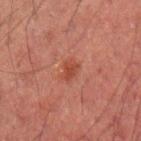Assessment: Part of a total-body skin-imaging series; this lesion was reviewed on a skin check and was not flagged for biopsy. Background: A male patient, aged 48–52. On the left upper arm. Cropped from a whole-body photographic skin survey; the tile spans about 15 mm.